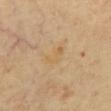This lesion was catalogued during total-body skin photography and was not selected for biopsy.
This image is a 15 mm lesion crop taken from a total-body photograph.
Longest diameter approximately 3 mm.
The subject is in their 60s.
The lesion is on the front of the torso.